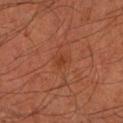The lesion was tiled from a total-body skin photograph and was not biopsied. Longest diameter approximately 1.5 mm. A lesion tile, about 15 mm wide, cut from a 3D total-body photograph. The subject is a male in their 60s. From the left forearm.